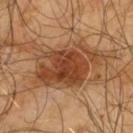follow-up=imaged on a skin check; not biopsied
imaging modality=total-body-photography crop, ~15 mm field of view
body site=the upper back
subject=male, approximately 65 years of age
lighting=cross-polarized